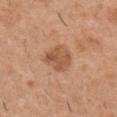Captured during whole-body skin photography for melanoma surveillance; the lesion was not biopsied. This image is a 15 mm lesion crop taken from a total-body photograph. A male subject about 40 years old. From the right upper arm.